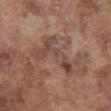Impression: Captured during whole-body skin photography for melanoma surveillance; the lesion was not biopsied. Background: On the chest. The lesion's longest dimension is about 7 mm. Captured under white-light illumination. The lesion-visualizer software estimated an average lesion color of about L≈46 a*≈19 b*≈24 (CIELAB), a lesion–skin lightness drop of about 8, and a normalized border contrast of about 6.5. The analysis additionally found a within-lesion color-variation index near 6/10. And it measured an automated nevus-likeness rating near 0 out of 100 and a detector confidence of about 70 out of 100 that the crop contains a lesion. A 15 mm close-up tile from a total-body photography series done for melanoma screening. The patient is a male in their mid-70s.